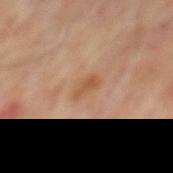Imaged during a routine full-body skin examination; the lesion was not biopsied and no histopathology is available. This image is a 15 mm lesion crop taken from a total-body photograph. Imaged with cross-polarized lighting. A male patient, roughly 65 years of age. The lesion is on the mid back. Automated image analysis of the tile measured a lesion area of about 4.5 mm², an eccentricity of roughly 0.8, and a shape-asymmetry score of about 0.25 (0 = symmetric). The analysis additionally found a nevus-likeness score of about 0/100 and a lesion-detection confidence of about 100/100.